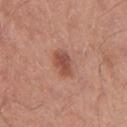Clinical impression:
Imaged during a routine full-body skin examination; the lesion was not biopsied and no histopathology is available.
Background:
The subject is a male aged 28 to 32. Imaged with white-light lighting. The lesion is on the right upper arm. Longest diameter approximately 3 mm. A roughly 15 mm field-of-view crop from a total-body skin photograph.